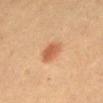<record>
  <biopsy_status>not biopsied; imaged during a skin examination</biopsy_status>
  <lighting>cross-polarized</lighting>
  <image>
    <source>total-body photography crop</source>
    <field_of_view_mm>15</field_of_view_mm>
  </image>
  <lesion_size>
    <long_diameter_mm_approx>3.0</long_diameter_mm_approx>
  </lesion_size>
  <patient>
    <sex>female</sex>
    <age_approx>40</age_approx>
  </patient>
  <site>abdomen</site>
</record>